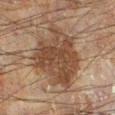• notes — total-body-photography surveillance lesion; no biopsy
• size — ~7 mm (longest diameter)
• imaging modality — 15 mm crop, total-body photography
• subject — male, aged 58 to 62
• site — the left leg
• illumination — cross-polarized
• TBP lesion metrics — a mean CIELAB color near L≈33 a*≈14 b*≈23 and a normalized border contrast of about 9; a border-irregularity rating of about 3.5/10, a within-lesion color-variation index near 4/10, and radial color variation of about 1.5